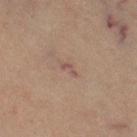biopsy status: imaged on a skin check; not biopsied | tile lighting: cross-polarized illumination | subject: female, aged around 70 | location: the left thigh | lesion size: about 2.5 mm | image: total-body-photography crop, ~15 mm field of view.